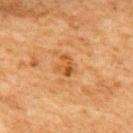Q: What is the lesion's diameter?
A: ~2.5 mm (longest diameter)
Q: Illumination type?
A: cross-polarized
Q: Lesion location?
A: the upper back
Q: What kind of image is this?
A: total-body-photography crop, ~15 mm field of view
Q: Automated lesion metrics?
A: an area of roughly 4 mm², a shape eccentricity near 0.65, and a symmetry-axis asymmetry near 0.35; border irregularity of about 3.5 on a 0–10 scale, a within-lesion color-variation index near 5/10, and radial color variation of about 2; a nevus-likeness score of about 15/100 and a lesion-detection confidence of about 100/100
Q: Patient demographics?
A: female, aged 58–62